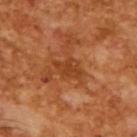Impression:
Captured during whole-body skin photography for melanoma surveillance; the lesion was not biopsied.
Image and clinical context:
The tile uses cross-polarized illumination. A 15 mm close-up tile from a total-body photography series done for melanoma screening. The subject is a male aged 63–67. The recorded lesion diameter is about 3.5 mm.